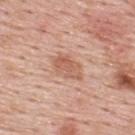Findings:
– notes — no biopsy performed (imaged during a skin exam)
– image — total-body-photography crop, ~15 mm field of view
– lighting — white-light
– size — ~4 mm (longest diameter)
– site — the upper back
– subject — male, approximately 55 years of age